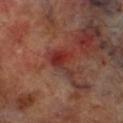Q: Was this lesion biopsied?
A: no biopsy performed (imaged during a skin exam)
Q: What did automated image analysis measure?
A: a footprint of about 7 mm² and a shape eccentricity near 0.75; border irregularity of about 6.5 on a 0–10 scale, internal color variation of about 4 on a 0–10 scale, and radial color variation of about 1; lesion-presence confidence of about 90/100
Q: How was this image acquired?
A: ~15 mm tile from a whole-body skin photo
Q: Lesion location?
A: the leg
Q: Patient demographics?
A: male, aged around 70
Q: What is the lesion's diameter?
A: ~4.5 mm (longest diameter)
Q: How was the tile lit?
A: cross-polarized